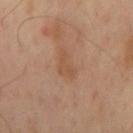{
  "biopsy_status": "not biopsied; imaged during a skin examination",
  "automated_metrics": {
    "area_mm2_approx": 4.5,
    "shape_asymmetry": 0.45,
    "cielab_L": 48,
    "cielab_a": 19,
    "cielab_b": 30,
    "vs_skin_darker_L": 6.0,
    "vs_skin_contrast_norm": 5.5,
    "border_irregularity_0_10": 5.0,
    "color_variation_0_10": 1.5,
    "peripheral_color_asymmetry": 0.5
  },
  "site": "mid back",
  "image": {
    "source": "total-body photography crop",
    "field_of_view_mm": 15
  },
  "patient": {
    "sex": "male",
    "age_approx": 65
  },
  "lighting": "cross-polarized"
}